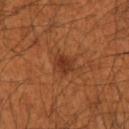A male patient aged approximately 35.
From the left forearm.
This image is a 15 mm lesion crop taken from a total-body photograph.